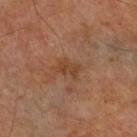The lesion was tiled from a total-body skin photograph and was not biopsied. A lesion tile, about 15 mm wide, cut from a 3D total-body photograph. The patient is a male approximately 70 years of age. The lesion is on the right lower leg.